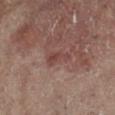notes: total-body-photography surveillance lesion; no biopsy
image source: 15 mm crop, total-body photography
body site: the left leg
lesion size: ~3 mm (longest diameter)
patient: female, aged approximately 80
lighting: cross-polarized illumination